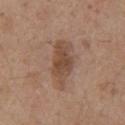Captured during whole-body skin photography for melanoma surveillance; the lesion was not biopsied.
A male patient, approximately 55 years of age.
Automated image analysis of the tile measured an eccentricity of roughly 0.9 and a shape-asymmetry score of about 0.25 (0 = symmetric). The software also gave a lesion color around L≈48 a*≈17 b*≈28 in CIELAB, about 9 CIELAB-L* units darker than the surrounding skin, and a lesion-to-skin contrast of about 7 (normalized; higher = more distinct). The software also gave a border-irregularity rating of about 3/10, a within-lesion color-variation index near 3.5/10, and a peripheral color-asymmetry measure near 1.
Captured under white-light illumination.
A lesion tile, about 15 mm wide, cut from a 3D total-body photograph.
About 6.5 mm across.
On the chest.